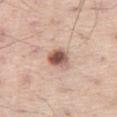This lesion was catalogued during total-body skin photography and was not selected for biopsy.
The total-body-photography lesion software estimated a nevus-likeness score of about 95/100 and lesion-presence confidence of about 100/100.
A male patient in their mid- to late 50s.
The lesion is located on the right thigh.
A roughly 15 mm field-of-view crop from a total-body skin photograph.
Approximately 2.5 mm at its widest.